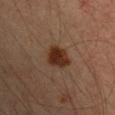This lesion was catalogued during total-body skin photography and was not selected for biopsy. Automated image analysis of the tile measured a footprint of about 8 mm², an eccentricity of roughly 0.45, and two-axis asymmetry of about 0.15. The analysis additionally found an average lesion color of about L≈28 a*≈18 b*≈26 (CIELAB), about 11 CIELAB-L* units darker than the surrounding skin, and a normalized border contrast of about 11. It also reported internal color variation of about 3 on a 0–10 scale and a peripheral color-asymmetry measure near 1. And it measured a nevus-likeness score of about 100/100. Cropped from a total-body skin-imaging series; the visible field is about 15 mm. The recorded lesion diameter is about 3.5 mm. The patient is a male in their mid-30s. The lesion is on the right upper arm. Imaged with cross-polarized lighting.